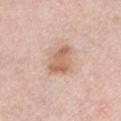| field | value |
|---|---|
| biopsy status | imaged on a skin check; not biopsied |